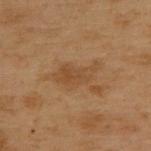follow-up = imaged on a skin check; not biopsied
image = 15 mm crop, total-body photography
anatomic site = the upper back
size = ≈5 mm
patient = male, aged 53 to 57